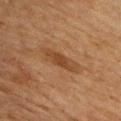The lesion was tiled from a total-body skin photograph and was not biopsied.
A male patient, aged approximately 45.
Located on the chest.
About 5 mm across.
A region of skin cropped from a whole-body photographic capture, roughly 15 mm wide.
The lesion-visualizer software estimated a lesion area of about 7 mm², a shape eccentricity near 0.95, and a shape-asymmetry score of about 0.25 (0 = symmetric). The software also gave a lesion color around L≈36 a*≈18 b*≈30 in CIELAB and about 7 CIELAB-L* units darker than the surrounding skin. The software also gave a border-irregularity index near 3.5/10. It also reported a classifier nevus-likeness of about 35/100 and a detector confidence of about 100 out of 100 that the crop contains a lesion.
The tile uses cross-polarized illumination.